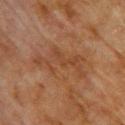follow-up: catalogued during a skin exam; not biopsied
lighting: cross-polarized
automated metrics: a footprint of about 12 mm² and an outline eccentricity of about 0.9 (0 = round, 1 = elongated); a lesion–skin lightness drop of about 5 and a normalized border contrast of about 5.5; a classifier nevus-likeness of about 0/100 and a lesion-detection confidence of about 100/100
body site: the upper back
diameter: about 7 mm
image: 15 mm crop, total-body photography
subject: female, roughly 80 years of age